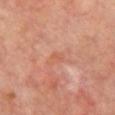Clinical impression: Part of a total-body skin-imaging series; this lesion was reviewed on a skin check and was not flagged for biopsy. Image and clinical context: The patient is a female roughly 50 years of age. Cropped from a total-body skin-imaging series; the visible field is about 15 mm. From the chest.